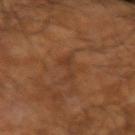This lesion was catalogued during total-body skin photography and was not selected for biopsy. This is a cross-polarized tile. The total-body-photography lesion software estimated an outline eccentricity of about 0.95 (0 = round, 1 = elongated) and a symmetry-axis asymmetry near 0.6. The software also gave a border-irregularity index near 6.5/10 and a peripheral color-asymmetry measure near 0.5. A male subject aged around 65. A roughly 15 mm field-of-view crop from a total-body skin photograph.